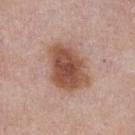Assessment:
Imaged during a routine full-body skin examination; the lesion was not biopsied and no histopathology is available.
Background:
A male subject, about 55 years old. The tile uses white-light illumination. Measured at roughly 6 mm in maximum diameter. This image is a 15 mm lesion crop taken from a total-body photograph. From the chest.